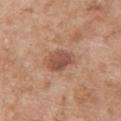Imaged during a routine full-body skin examination; the lesion was not biopsied and no histopathology is available. The patient is a female aged 58–62. A close-up tile cropped from a whole-body skin photograph, about 15 mm across. On the upper back.